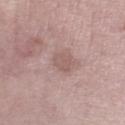Recorded during total-body skin imaging; not selected for excision or biopsy. The subject is a female in their 60s. Approximately 3 mm at its widest. A 15 mm crop from a total-body photograph taken for skin-cancer surveillance. The lesion is located on the right lower leg. The tile uses white-light illumination.